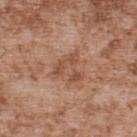About 4 mm across.
The lesion is located on the upper back.
This is a white-light tile.
A roughly 15 mm field-of-view crop from a total-body skin photograph.
The patient is a male about 55 years old.
Automated tile analysis of the lesion measured a footprint of about 8.5 mm², an eccentricity of roughly 0.25, and a shape-asymmetry score of about 0.55 (0 = symmetric). It also reported a lesion–skin lightness drop of about 8. The software also gave a border-irregularity index near 6.5/10.